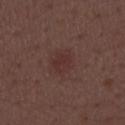size — ~3 mm (longest diameter) | patient — male, about 50 years old | image source — total-body-photography crop, ~15 mm field of view | tile lighting — white-light.